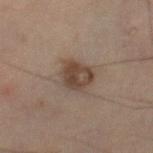Q: Who is the patient?
A: male, aged around 60
Q: How was this image acquired?
A: ~15 mm crop, total-body skin-cancer survey
Q: What is the lesion's diameter?
A: ~3.5 mm (longest diameter)
Q: Automated lesion metrics?
A: about 9 CIELAB-L* units darker than the surrounding skin; border irregularity of about 2 on a 0–10 scale, a within-lesion color-variation index near 4.5/10, and radial color variation of about 1.5
Q: What is the anatomic site?
A: the leg